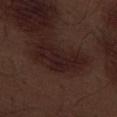patient:
  sex: male
  age_approx: 70
site: left thigh
image:
  source: total-body photography crop
  field_of_view_mm: 15